A 15 mm close-up tile from a total-body photography series done for melanoma screening · located on the chest · a female subject aged 48 to 52:
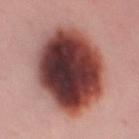The lesion was biopsied, and histopathology showed a dysplastic (Clark) nevus.